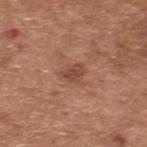Automated image analysis of the tile measured an area of roughly 3.5 mm², an eccentricity of roughly 0.65, and two-axis asymmetry of about 0.4. And it measured about 9 CIELAB-L* units darker than the surrounding skin and a lesion-to-skin contrast of about 7 (normalized; higher = more distinct). The software also gave an automated nevus-likeness rating near 0 out of 100 and a detector confidence of about 100 out of 100 that the crop contains a lesion.
The tile uses white-light illumination.
Located on the upper back.
The patient is a male in their mid-60s.
A roughly 15 mm field-of-view crop from a total-body skin photograph.
Measured at roughly 2.5 mm in maximum diameter.